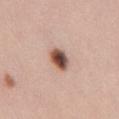Recorded during total-body skin imaging; not selected for excision or biopsy. This is a white-light tile. Approximately 3 mm at its widest. A female patient roughly 25 years of age. A 15 mm crop from a total-body photograph taken for skin-cancer surveillance. The lesion is located on the front of the torso.